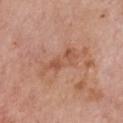Impression:
The lesion was tiled from a total-body skin photograph and was not biopsied.
Clinical summary:
About 5 mm across. The total-body-photography lesion software estimated a footprint of about 7.5 mm², a shape eccentricity near 0.95, and a shape-asymmetry score of about 0.45 (0 = symmetric). And it measured a lesion color around L≈54 a*≈24 b*≈32 in CIELAB and a lesion–skin lightness drop of about 7. A 15 mm close-up extracted from a 3D total-body photography capture. On the chest. Captured under white-light illumination. A female subject aged approximately 65.